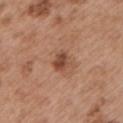notes = catalogued during a skin exam; not biopsied | body site = the left upper arm | patient = male, approximately 55 years of age | lesion size = ≈2.5 mm | image-analysis metrics = a border-irregularity index near 1.5/10, internal color variation of about 2.5 on a 0–10 scale, and a peripheral color-asymmetry measure near 1 | image source = ~15 mm crop, total-body skin-cancer survey.